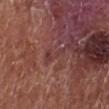Assessment:
The lesion was photographed on a routine skin check and not biopsied; there is no pathology result.
Context:
A 15 mm close-up tile from a total-body photography series done for melanoma screening. Located on the left lower leg. The tile uses white-light illumination. Measured at roughly 3.5 mm in maximum diameter. A male subject, in their mid-70s.